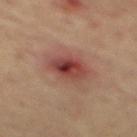Notes:
– notes · imaged on a skin check; not biopsied
– subject · male, in their mid-60s
– automated lesion analysis · a footprint of about 10 mm², an outline eccentricity of about 0.5 (0 = round, 1 = elongated), and a symmetry-axis asymmetry near 0.15; a mean CIELAB color near L≈38 a*≈24 b*≈23, roughly 10 lightness units darker than nearby skin, and a lesion-to-skin contrast of about 9 (normalized; higher = more distinct); border irregularity of about 1.5 on a 0–10 scale and a color-variation rating of about 8/10; an automated nevus-likeness rating near 0 out of 100 and lesion-presence confidence of about 100/100
– location · the mid back
– diameter · ~4 mm (longest diameter)
– image · 15 mm crop, total-body photography
– tile lighting · cross-polarized